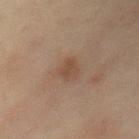Q: Is there a histopathology result?
A: total-body-photography surveillance lesion; no biopsy
Q: Who is the patient?
A: female, about 70 years old
Q: Lesion size?
A: ~3 mm (longest diameter)
Q: What lighting was used for the tile?
A: cross-polarized illumination
Q: What is the anatomic site?
A: the chest
Q: What is the imaging modality?
A: ~15 mm tile from a whole-body skin photo